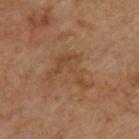Q: Is there a histopathology result?
A: total-body-photography surveillance lesion; no biopsy
Q: How large is the lesion?
A: ≈5.5 mm
Q: Automated lesion metrics?
A: an outline eccentricity of about 0.65 (0 = round, 1 = elongated) and a shape-asymmetry score of about 0.8 (0 = symmetric); a mean CIELAB color near L≈47 a*≈20 b*≈34, about 6 CIELAB-L* units darker than the surrounding skin, and a lesion-to-skin contrast of about 5 (normalized; higher = more distinct); an automated nevus-likeness rating near 0 out of 100 and lesion-presence confidence of about 100/100
Q: What is the anatomic site?
A: the upper back
Q: How was this image acquired?
A: ~15 mm tile from a whole-body skin photo
Q: What lighting was used for the tile?
A: cross-polarized illumination